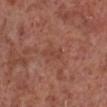| field | value |
|---|---|
| follow-up | no biopsy performed (imaged during a skin exam) |
| location | the leg |
| patient | male, about 55 years old |
| image source | ~15 mm crop, total-body skin-cancer survey |